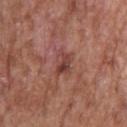The lesion was photographed on a routine skin check and not biopsied; there is no pathology result. Measured at roughly 3 mm in maximum diameter. The lesion is on the upper back. A lesion tile, about 15 mm wide, cut from a 3D total-body photograph. The tile uses white-light illumination. The patient is a female in their mid- to late 70s.